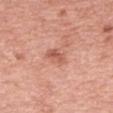biopsy status: total-body-photography surveillance lesion; no biopsy
imaging modality: ~15 mm tile from a whole-body skin photo
TBP lesion metrics: a mean CIELAB color near L≈58 a*≈27 b*≈31, a lesion–skin lightness drop of about 10, and a normalized border contrast of about 6.5; a classifier nevus-likeness of about 15/100 and a detector confidence of about 100 out of 100 that the crop contains a lesion
patient: female, approximately 40 years of age
body site: the arm